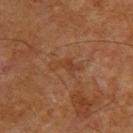The lesion was tiled from a total-body skin photograph and was not biopsied.
Longest diameter approximately 3.5 mm.
Cropped from a total-body skin-imaging series; the visible field is about 15 mm.
Captured under cross-polarized illumination.
A male subject, in their mid- to late 60s.
The lesion is on the upper back.